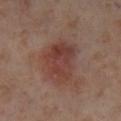Part of a total-body skin-imaging series; this lesion was reviewed on a skin check and was not flagged for biopsy. Imaged with cross-polarized lighting. The patient is a female in their mid-50s. A close-up tile cropped from a whole-body skin photograph, about 15 mm across. Located on the leg. The recorded lesion diameter is about 5.5 mm.